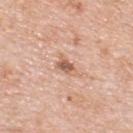Case summary:
– follow-up — no biopsy performed (imaged during a skin exam)
– patient — male, aged around 80
– body site — the back
– image — 15 mm crop, total-body photography